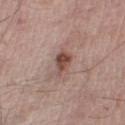workup: no biopsy performed (imaged during a skin exam) | lighting: white-light | imaging modality: ~15 mm tile from a whole-body skin photo | size: ≈3 mm | body site: the left lower leg | patient: male, in their mid- to late 50s | automated metrics: an area of roughly 4 mm², a shape eccentricity near 0.85, and a shape-asymmetry score of about 0.35 (0 = symmetric); a lesion color around L≈47 a*≈20 b*≈24 in CIELAB, about 13 CIELAB-L* units darker than the surrounding skin, and a lesion-to-skin contrast of about 9.5 (normalized; higher = more distinct); a border-irregularity index near 3.5/10 and peripheral color asymmetry of about 1; an automated nevus-likeness rating near 80 out of 100 and lesion-presence confidence of about 100/100.